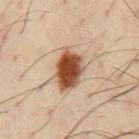Findings:
* follow-up · imaged on a skin check; not biopsied
* image source · ~15 mm crop, total-body skin-cancer survey
* anatomic site · the chest
* subject · male, in their 40s